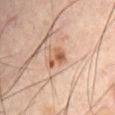Q: Is there a histopathology result?
A: imaged on a skin check; not biopsied
Q: What is the anatomic site?
A: the abdomen
Q: Patient demographics?
A: male, about 65 years old
Q: How large is the lesion?
A: ≈3 mm
Q: What lighting was used for the tile?
A: cross-polarized
Q: What kind of image is this?
A: 15 mm crop, total-body photography